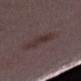workup — total-body-photography surveillance lesion; no biopsy
image — ~15 mm tile from a whole-body skin photo
site — the right lower leg
patient — female, aged around 30